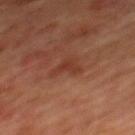The lesion was tiled from a total-body skin photograph and was not biopsied.
This is a cross-polarized tile.
A lesion tile, about 15 mm wide, cut from a 3D total-body photograph.
Located on the mid back.
Longest diameter approximately 3 mm.
The subject is a male roughly 65 years of age.